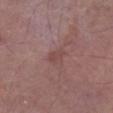- anatomic site: the right lower leg
- subject: male, in their 80s
- lesion diameter: about 2.5 mm
- image source: ~15 mm tile from a whole-body skin photo
- automated metrics: a normalized lesion–skin contrast near 5; a color-variation rating of about 1.5/10 and a peripheral color-asymmetry measure near 0.5; a classifier nevus-likeness of about 0/100